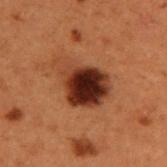Q: Was this lesion biopsied?
A: no biopsy performed (imaged during a skin exam)
Q: How was the tile lit?
A: cross-polarized illumination
Q: What is the imaging modality?
A: ~15 mm crop, total-body skin-cancer survey
Q: Where on the body is the lesion?
A: the upper back
Q: Who is the patient?
A: male, aged approximately 50
Q: Automated lesion metrics?
A: an area of roughly 18 mm², an outline eccentricity of about 0.7 (0 = round, 1 = elongated), and a shape-asymmetry score of about 0.35 (0 = symmetric); an average lesion color of about L≈26 a*≈21 b*≈25 (CIELAB), roughly 15 lightness units darker than nearby skin, and a normalized border contrast of about 14.5; a border-irregularity index near 3.5/10, a color-variation rating of about 9/10, and radial color variation of about 2.5; a classifier nevus-likeness of about 80/100 and lesion-presence confidence of about 100/100